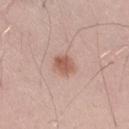biopsy_status: not biopsied; imaged during a skin examination
site: right thigh
patient:
  sex: male
  age_approx: 55
automated_metrics:
  area_mm2_approx: 5.0
  eccentricity: 0.6
  shape_asymmetry: 0.15
  color_variation_0_10: 3.0
  lesion_detection_confidence_0_100: 100
image:
  source: total-body photography crop
  field_of_view_mm: 15